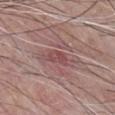The lesion was tiled from a total-body skin photograph and was not biopsied.
The patient is a male in their mid- to late 80s or older.
This is a white-light tile.
From the chest.
Cropped from a total-body skin-imaging series; the visible field is about 15 mm.
Measured at roughly 2.5 mm in maximum diameter.